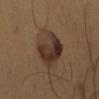notes = no biopsy performed (imaged during a skin exam) | anatomic site = the left upper arm | subject = male, about 50 years old | acquisition = ~15 mm crop, total-body skin-cancer survey | size = ~5 mm (longest diameter).